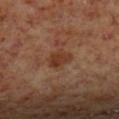{
  "patient": {
    "sex": "male",
    "age_approx": 60
  },
  "site": "left lower leg",
  "image": {
    "source": "total-body photography crop",
    "field_of_view_mm": 15
  },
  "lesion_size": {
    "long_diameter_mm_approx": 3.0
  },
  "lighting": "cross-polarized"
}